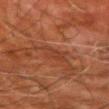workup: imaged on a skin check; not biopsied | image source: ~15 mm crop, total-body skin-cancer survey | subject: male, aged around 80 | tile lighting: cross-polarized | lesion diameter: ~3 mm (longest diameter) | site: the left upper arm.